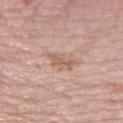biopsy status: catalogued during a skin exam; not biopsied.